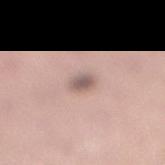The lesion is located on the right lower leg. The lesion's longest dimension is about 2 mm. A female patient, roughly 40 years of age. The tile uses white-light illumination. A roughly 15 mm field-of-view crop from a total-body skin photograph.